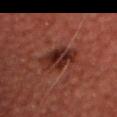No biopsy was performed on this lesion — it was imaged during a full skin examination and was not determined to be concerning.